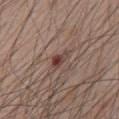{
  "automated_metrics": {
    "vs_skin_darker_L": 11.0,
    "vs_skin_contrast_norm": 8.5,
    "border_irregularity_0_10": 3.0,
    "color_variation_0_10": 4.0,
    "peripheral_color_asymmetry": 1.0,
    "nevus_likeness_0_100": 10,
    "lesion_detection_confidence_0_100": 100
  },
  "lesion_size": {
    "long_diameter_mm_approx": 2.5
  },
  "image": {
    "source": "total-body photography crop",
    "field_of_view_mm": 15
  },
  "site": "chest",
  "patient": {
    "sex": "male",
    "age_approx": 65
  }
}